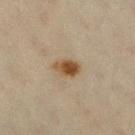workup — imaged on a skin check; not biopsied | subject — female, in their mid- to late 60s | image source — 15 mm crop, total-body photography | location — the right lower leg.